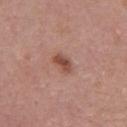The lesion was photographed on a routine skin check and not biopsied; there is no pathology result. This is a white-light tile. A 15 mm close-up extracted from a 3D total-body photography capture. A female patient, about 50 years old. The lesion is on the front of the torso. The lesion's longest dimension is about 2.5 mm.